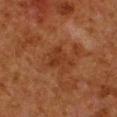  lesion_size:
    long_diameter_mm_approx: 3.0
  patient:
    sex: male
    age_approx: 80
  image:
    source: total-body photography crop
    field_of_view_mm: 15
  lighting: cross-polarized
  site: left lower leg
  automated_metrics:
    cielab_L: 27
    cielab_a: 21
    cielab_b: 28
    vs_skin_darker_L: 5.0
    nevus_likeness_0_100: 0
    lesion_detection_confidence_0_100: 100